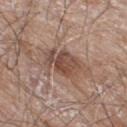biopsy status: total-body-photography surveillance lesion; no biopsy | size: about 5 mm | patient: male, aged 58 to 62 | location: the right thigh | illumination: white-light | image source: 15 mm crop, total-body photography.